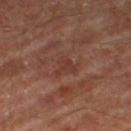workup=no biopsy performed (imaged during a skin exam)